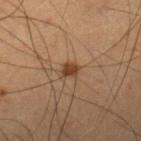Recorded during total-body skin imaging; not selected for excision or biopsy.
Located on the leg.
A region of skin cropped from a whole-body photographic capture, roughly 15 mm wide.
Measured at roughly 2 mm in maximum diameter.
This is a cross-polarized tile.
A male patient, about 65 years old.